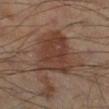biopsy status = total-body-photography surveillance lesion; no biopsy | lighting = cross-polarized illumination | imaging modality = total-body-photography crop, ~15 mm field of view | location = the right lower leg | subject = male, in their mid- to late 50s | diameter = ~6 mm (longest diameter).